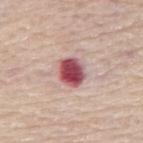Clinical impression:
Recorded during total-body skin imaging; not selected for excision or biopsy.
Clinical summary:
Imaged with white-light lighting. The total-body-photography lesion software estimated an eccentricity of roughly 0.6. And it measured an average lesion color of about L≈52 a*≈30 b*≈19 (CIELAB), roughly 20 lightness units darker than nearby skin, and a normalized border contrast of about 13. And it measured a classifier nevus-likeness of about 0/100 and lesion-presence confidence of about 100/100. The lesion is on the back. A male patient aged 73 to 77. Longest diameter approximately 3.5 mm. Cropped from a total-body skin-imaging series; the visible field is about 15 mm.